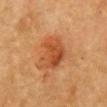– biopsy status — total-body-photography surveillance lesion; no biopsy
– lesion diameter — ~5 mm (longest diameter)
– imaging modality — ~15 mm crop, total-body skin-cancer survey
– subject — female, aged around 55
– site — the chest
– image-analysis metrics — a lesion area of about 12 mm², an eccentricity of roughly 0.75, and a symmetry-axis asymmetry near 0.3; a lesion color around L≈44 a*≈25 b*≈36 in CIELAB, roughly 9 lightness units darker than nearby skin, and a lesion-to-skin contrast of about 7.5 (normalized; higher = more distinct)